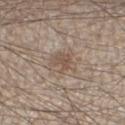Part of a total-body skin-imaging series; this lesion was reviewed on a skin check and was not flagged for biopsy. The subject is a male approximately 45 years of age. A 15 mm crop from a total-body photograph taken for skin-cancer surveillance. Imaged with white-light lighting. Automated image analysis of the tile measured an area of roughly 7 mm² and an outline eccentricity of about 0.4 (0 = round, 1 = elongated). The lesion is on the left lower leg.